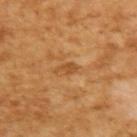| field | value |
|---|---|
| notes | no biopsy performed (imaged during a skin exam) |
| lighting | cross-polarized |
| image | total-body-photography crop, ~15 mm field of view |
| subject | male, in their mid-60s |
| lesion size | ≈2.5 mm |
| automated metrics | an area of roughly 2.5 mm², an outline eccentricity of about 0.85 (0 = round, 1 = elongated), and a shape-asymmetry score of about 0.4 (0 = symmetric); a mean CIELAB color near L≈49 a*≈23 b*≈41, roughly 8 lightness units darker than nearby skin, and a normalized lesion–skin contrast near 6.5 |